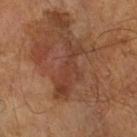Assessment: Imaged during a routine full-body skin examination; the lesion was not biopsied and no histopathology is available.